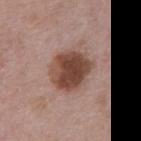Imaged during a routine full-body skin examination; the lesion was not biopsied and no histopathology is available. A male patient, aged around 80. A 15 mm crop from a total-body photograph taken for skin-cancer surveillance. The lesion is on the chest. Imaged with white-light lighting. An algorithmic analysis of the crop reported a lesion color around L≈45 a*≈20 b*≈25 in CIELAB, roughly 15 lightness units darker than nearby skin, and a normalized border contrast of about 11. It also reported a border-irregularity rating of about 1.5/10, internal color variation of about 5 on a 0–10 scale, and a peripheral color-asymmetry measure near 1.5. The software also gave a nevus-likeness score of about 65/100.